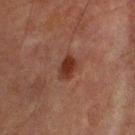biopsy status — imaged on a skin check; not biopsied | location — the arm | imaging modality — ~15 mm tile from a whole-body skin photo | patient — male, about 80 years old.